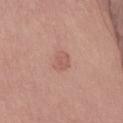follow-up=total-body-photography surveillance lesion; no biopsy
location=the chest
lesion size=≈3 mm
illumination=white-light
subject=male, approximately 65 years of age
automated lesion analysis=an eccentricity of roughly 0.65 and a symmetry-axis asymmetry near 0.25; a border-irregularity rating of about 2/10, a color-variation rating of about 2/10, and radial color variation of about 0.5; an automated nevus-likeness rating near 20 out of 100 and a lesion-detection confidence of about 100/100
acquisition=15 mm crop, total-body photography